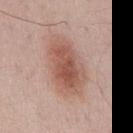Q: Is there a histopathology result?
A: total-body-photography surveillance lesion; no biopsy
Q: Where on the body is the lesion?
A: the mid back
Q: Illumination type?
A: white-light illumination
Q: How large is the lesion?
A: about 8 mm
Q: Who is the patient?
A: male, about 50 years old
Q: What kind of image is this?
A: 15 mm crop, total-body photography